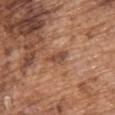follow-up: catalogued during a skin exam; not biopsied
patient: male, aged 73–77
acquisition: ~15 mm tile from a whole-body skin photo
body site: the upper back